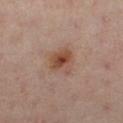Notes:
- workup · imaged on a skin check; not biopsied
- subject · female, aged 38 to 42
- acquisition · ~15 mm tile from a whole-body skin photo
- anatomic site · the left leg
- diameter · about 3.5 mm
- illumination · cross-polarized
- image-analysis metrics · an eccentricity of roughly 0.3; a border-irregularity rating of about 2.5/10 and radial color variation of about 2